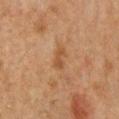* follow-up — catalogued during a skin exam; not biopsied
* site — the chest
* lesion size — ~2.5 mm (longest diameter)
* image source — 15 mm crop, total-body photography
* lighting — cross-polarized
* image-analysis metrics — a mean CIELAB color near L≈40 a*≈18 b*≈31, roughly 7 lightness units darker than nearby skin, and a normalized border contrast of about 6.5; a border-irregularity rating of about 4.5/10 and a within-lesion color-variation index near 0/10
* patient — male, approximately 50 years of age